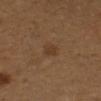{
  "image": {
    "source": "total-body photography crop",
    "field_of_view_mm": 15
  },
  "automated_metrics": {
    "border_irregularity_0_10": 2.0,
    "peripheral_color_asymmetry": 0.5
  },
  "lighting": "cross-polarized",
  "site": "right lower leg",
  "patient": {
    "sex": "female",
    "age_approx": 55
  },
  "lesion_size": {
    "long_diameter_mm_approx": 1.5
  }
}